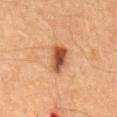follow-up: no biopsy performed (imaged during a skin exam)
image: ~15 mm tile from a whole-body skin photo
patient: male, in their mid- to late 60s
site: the mid back